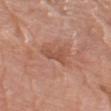<case>
<biopsy_status>not biopsied; imaged during a skin examination</biopsy_status>
<patient>
  <sex>male</sex>
  <age_approx>80</age_approx>
</patient>
<site>arm</site>
<image>
  <source>total-body photography crop</source>
  <field_of_view_mm>15</field_of_view_mm>
</image>
<lighting>white-light</lighting>
<lesion_size>
  <long_diameter_mm_approx>3.5</long_diameter_mm_approx>
</lesion_size>
</case>